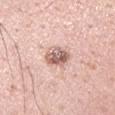Case summary:
• follow-up: imaged on a skin check; not biopsied
• subject: male, in their mid- to late 30s
• imaging modality: ~15 mm tile from a whole-body skin photo
• tile lighting: white-light illumination
• location: the left upper arm
• image-analysis metrics: a footprint of about 6 mm² and an outline eccentricity of about 0.4 (0 = round, 1 = elongated); a lesion-to-skin contrast of about 9.5 (normalized; higher = more distinct); border irregularity of about 2.5 on a 0–10 scale, internal color variation of about 7.5 on a 0–10 scale, and peripheral color asymmetry of about 2.5
• diameter: ~3 mm (longest diameter)